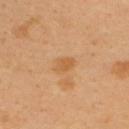Case summary:
* biopsy status · catalogued during a skin exam; not biopsied
* subject · male, aged 38–42
* site · the upper back
* image · 15 mm crop, total-body photography